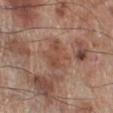The lesion was tiled from a total-body skin photograph and was not biopsied.
A region of skin cropped from a whole-body photographic capture, roughly 15 mm wide.
A male patient, aged 68–72.
On the right lower leg.